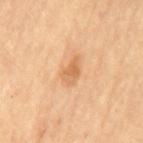No biopsy was performed on this lesion — it was imaged during a full skin examination and was not determined to be concerning.
A region of skin cropped from a whole-body photographic capture, roughly 15 mm wide.
The patient is a male roughly 85 years of age or older.
From the back.
The total-body-photography lesion software estimated an area of roughly 4 mm², an eccentricity of roughly 0.8, and a shape-asymmetry score of about 0.4 (0 = symmetric). And it measured a border-irregularity index near 3.5/10, a color-variation rating of about 2.5/10, and peripheral color asymmetry of about 0.5.